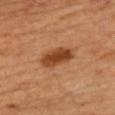Assessment:
The lesion was photographed on a routine skin check and not biopsied; there is no pathology result.
Acquisition and patient details:
The lesion's longest dimension is about 4.5 mm. A male patient, about 40 years old. Cropped from a whole-body photographic skin survey; the tile spans about 15 mm. Located on the chest. Captured under cross-polarized illumination.